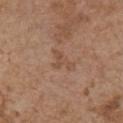Recorded during total-body skin imaging; not selected for excision or biopsy.
From the chest.
Cropped from a total-body skin-imaging series; the visible field is about 15 mm.
Automated image analysis of the tile measured an average lesion color of about L≈49 a*≈19 b*≈29 (CIELAB), about 6 CIELAB-L* units darker than the surrounding skin, and a normalized lesion–skin contrast near 5. The software also gave an automated nevus-likeness rating near 0 out of 100 and a lesion-detection confidence of about 100/100.
The subject is a female about 65 years old.
Imaged with white-light lighting.